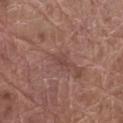The lesion was tiled from a total-body skin photograph and was not biopsied.
A male subject aged 78 to 82.
The lesion is on the right thigh.
A roughly 15 mm field-of-view crop from a total-body skin photograph.
Imaged with white-light lighting.
About 2.5 mm across.